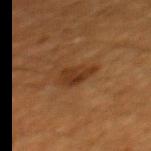Clinical impression:
Part of a total-body skin-imaging series; this lesion was reviewed on a skin check and was not flagged for biopsy.
Clinical summary:
From the back. A 15 mm close-up tile from a total-body photography series done for melanoma screening. The subject is a male in their 60s.